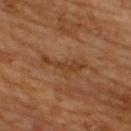  biopsy_status: not biopsied; imaged during a skin examination
  lighting: cross-polarized
  site: upper back
  automated_metrics:
    area_mm2_approx: 6.5
    shape_asymmetry: 0.55
    cielab_L: 39
    cielab_a: 21
    cielab_b: 33
    vs_skin_darker_L: 7.0
    vs_skin_contrast_norm: 6.0
    border_irregularity_0_10: 8.5
    color_variation_0_10: 1.5
  patient:
    sex: male
    age_approx: 65
  image:
    source: total-body photography crop
    field_of_view_mm: 15
  lesion_size:
    long_diameter_mm_approx: 5.5